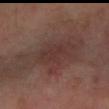Assessment: The lesion was photographed on a routine skin check and not biopsied; there is no pathology result. Background: The lesion is located on the arm. The lesion's longest dimension is about 8.5 mm. This is a cross-polarized tile. A female patient, approximately 55 years of age. A 15 mm crop from a total-body photograph taken for skin-cancer surveillance.